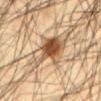Q: Was this lesion biopsied?
A: catalogued during a skin exam; not biopsied